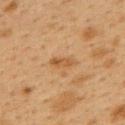Findings:
– follow-up: catalogued during a skin exam; not biopsied
– imaging modality: ~15 mm tile from a whole-body skin photo
– lesion size: ~3 mm (longest diameter)
– subject: female, roughly 40 years of age
– location: the back
– automated lesion analysis: a lesion area of about 3.5 mm² and a symmetry-axis asymmetry near 0.3; an average lesion color of about L≈45 a*≈18 b*≈34 (CIELAB), about 7 CIELAB-L* units darker than the surrounding skin, and a normalized border contrast of about 6.5
– tile lighting: cross-polarized illumination